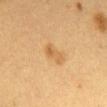Imaged during a routine full-body skin examination; the lesion was not biopsied and no histopathology is available. The total-body-photography lesion software estimated a shape eccentricity near 0.9 and a shape-asymmetry score of about 0.25 (0 = symmetric). The analysis additionally found lesion-presence confidence of about 100/100. A female patient approximately 40 years of age. A 15 mm close-up tile from a total-body photography series done for melanoma screening. This is a cross-polarized tile. The lesion is on the mid back.